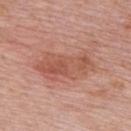The lesion was tiled from a total-body skin photograph and was not biopsied.
A male subject about 75 years old.
On the upper back.
Longest diameter approximately 7 mm.
A 15 mm crop from a total-body photograph taken for skin-cancer surveillance.